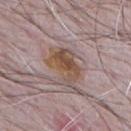Q: Was a biopsy performed?
A: imaged on a skin check; not biopsied
Q: How was this image acquired?
A: total-body-photography crop, ~15 mm field of view
Q: Where on the body is the lesion?
A: the mid back
Q: What are the patient's age and sex?
A: male, aged approximately 65
Q: What is the lesion's diameter?
A: ~4.5 mm (longest diameter)
Q: How was the tile lit?
A: white-light